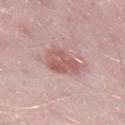Captured during whole-body skin photography for melanoma surveillance; the lesion was not biopsied.
Captured under white-light illumination.
The total-body-photography lesion software estimated an automated nevus-likeness rating near 45 out of 100 and a detector confidence of about 100 out of 100 that the crop contains a lesion.
The lesion is on the right lower leg.
Cropped from a total-body skin-imaging series; the visible field is about 15 mm.
The patient is a male about 50 years old.
Approximately 5 mm at its widest.